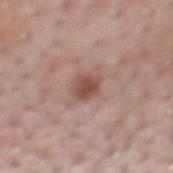automated lesion analysis=a footprint of about 5 mm², a shape eccentricity near 0.45, and a symmetry-axis asymmetry near 0.15; a border-irregularity index near 1.5/10, a within-lesion color-variation index near 2.5/10, and radial color variation of about 0.5
site=the mid back
lighting=white-light illumination
lesion size=~2.5 mm (longest diameter)
subject=male, aged 53–57
image source=~15 mm tile from a whole-body skin photo